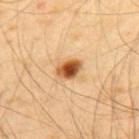Q: Was a biopsy performed?
A: imaged on a skin check; not biopsied
Q: Lesion location?
A: the upper back
Q: How large is the lesion?
A: ≈3 mm
Q: Illumination type?
A: cross-polarized illumination
Q: What are the patient's age and sex?
A: male, aged around 40
Q: What kind of image is this?
A: 15 mm crop, total-body photography